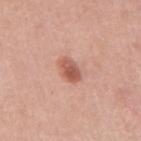Assessment:
The lesion was photographed on a routine skin check and not biopsied; there is no pathology result.